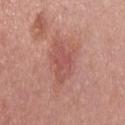  biopsy_status: not biopsied; imaged during a skin examination
  lighting: white-light
  patient:
    sex: male
    age_approx: 30
  lesion_size:
    long_diameter_mm_approx: 5.0
  image:
    source: total-body photography crop
    field_of_view_mm: 15